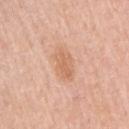Notes:
* follow-up: imaged on a skin check; not biopsied
* diameter: ≈3.5 mm
* automated metrics: a shape eccentricity near 0.8; a border-irregularity rating of about 2.5/10, internal color variation of about 1.5 on a 0–10 scale, and peripheral color asymmetry of about 0.5; a nevus-likeness score of about 10/100
* image source: total-body-photography crop, ~15 mm field of view
* subject: female, about 65 years old
* site: the left arm